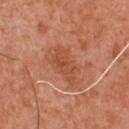follow-up = imaged on a skin check; not biopsied | imaging modality = total-body-photography crop, ~15 mm field of view | subject = male, aged 53 to 57 | body site = the front of the torso.